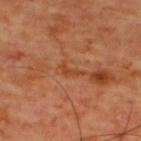Clinical impression: The lesion was tiled from a total-body skin photograph and was not biopsied. Clinical summary: Automated image analysis of the tile measured a lesion area of about 2.5 mm² and two-axis asymmetry of about 0.6. The software also gave a normalized lesion–skin contrast near 6. It also reported a classifier nevus-likeness of about 0/100 and a detector confidence of about 85 out of 100 that the crop contains a lesion. The tile uses cross-polarized illumination. A male subject, aged 58–62. The lesion's longest dimension is about 3 mm. A 15 mm close-up extracted from a 3D total-body photography capture. The lesion is located on the upper back.